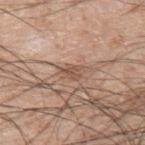{"biopsy_status": "not biopsied; imaged during a skin examination", "site": "upper back", "lighting": "white-light", "patient": {"sex": "male", "age_approx": 45}, "image": {"source": "total-body photography crop", "field_of_view_mm": 15}}